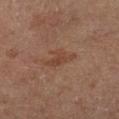The lesion was photographed on a routine skin check and not biopsied; there is no pathology result. A lesion tile, about 15 mm wide, cut from a 3D total-body photograph. The lesion is located on the left lower leg. Imaged with cross-polarized lighting. A male subject aged around 65.